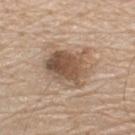Part of a total-body skin-imaging series; this lesion was reviewed on a skin check and was not flagged for biopsy.
A close-up tile cropped from a whole-body skin photograph, about 15 mm across.
An algorithmic analysis of the crop reported an area of roughly 17 mm², a shape eccentricity near 0.55, and a symmetry-axis asymmetry near 0.25. It also reported internal color variation of about 7 on a 0–10 scale and peripheral color asymmetry of about 2.5.
The lesion is located on the upper back.
About 5 mm across.
A male patient in their 80s.
The tile uses white-light illumination.